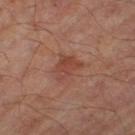No biopsy was performed on this lesion — it was imaged during a full skin examination and was not determined to be concerning.
The lesion is on the left thigh.
Approximately 3.5 mm at its widest.
A 15 mm close-up tile from a total-body photography series done for melanoma screening.
A male patient, aged around 65.